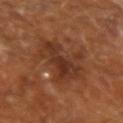Impression:
Imaged during a routine full-body skin examination; the lesion was not biopsied and no histopathology is available.
Context:
From the arm. A region of skin cropped from a whole-body photographic capture, roughly 15 mm wide. A male patient, aged approximately 65.